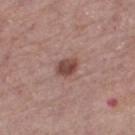| feature | finding |
|---|---|
| biopsy status | no biopsy performed (imaged during a skin exam) |
| size | about 2.5 mm |
| image | total-body-photography crop, ~15 mm field of view |
| patient | female, aged 63 to 67 |
| image-analysis metrics | a lesion area of about 5 mm² and a shape-asymmetry score of about 0.15 (0 = symmetric); a mean CIELAB color near L≈46 a*≈21 b*≈23, about 12 CIELAB-L* units darker than the surrounding skin, and a lesion-to-skin contrast of about 9 (normalized; higher = more distinct); a border-irregularity index near 1.5/10, a color-variation rating of about 3.5/10, and radial color variation of about 1 |
| site | the right thigh |